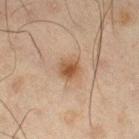Impression:
This lesion was catalogued during total-body skin photography and was not selected for biopsy.
Acquisition and patient details:
A lesion tile, about 15 mm wide, cut from a 3D total-body photograph. The patient is a male approximately 45 years of age. The lesion is on the right thigh. The tile uses cross-polarized illumination.